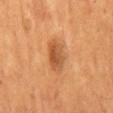Clinical impression: The lesion was tiled from a total-body skin photograph and was not biopsied. Context: A male patient roughly 55 years of age. Cropped from a whole-body photographic skin survey; the tile spans about 15 mm. The tile uses cross-polarized illumination. The lesion is on the back. The lesion-visualizer software estimated a lesion area of about 8.5 mm² and two-axis asymmetry of about 0.2. The analysis additionally found an average lesion color of about L≈50 a*≈24 b*≈38 (CIELAB), a lesion–skin lightness drop of about 10, and a lesion-to-skin contrast of about 7.5 (normalized; higher = more distinct). And it measured a border-irregularity index near 2/10, a within-lesion color-variation index near 4/10, and radial color variation of about 1. And it measured an automated nevus-likeness rating near 75 out of 100 and a lesion-detection confidence of about 100/100.